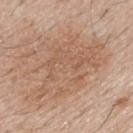The lesion was tiled from a total-body skin photograph and was not biopsied.
Located on the mid back.
A 15 mm crop from a total-body photograph taken for skin-cancer surveillance.
An algorithmic analysis of the crop reported border irregularity of about 8 on a 0–10 scale, a color-variation rating of about 3.5/10, and radial color variation of about 1.
A male subject, aged approximately 55.
Captured under white-light illumination.